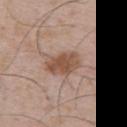Impression: Imaged during a routine full-body skin examination; the lesion was not biopsied and no histopathology is available. Context: On the chest. Approximately 4.5 mm at its widest. The patient is a male aged approximately 55. This image is a 15 mm lesion crop taken from a total-body photograph.